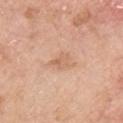  site: upper back
  image:
    source: total-body photography crop
    field_of_view_mm: 15
  patient:
    sex: male
    age_approx: 65